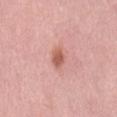workup: catalogued during a skin exam; not biopsied | imaging modality: ~15 mm tile from a whole-body skin photo | illumination: white-light illumination | anatomic site: the mid back | subject: female, aged 58 to 62.